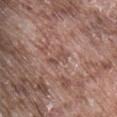image source: 15 mm crop, total-body photography; subject: male, in their mid-70s; body site: the abdomen.